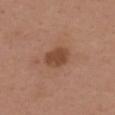Imaged during a routine full-body skin examination; the lesion was not biopsied and no histopathology is available. Imaged with white-light lighting. A male subject aged around 40. Longest diameter approximately 3 mm. A 15 mm close-up tile from a total-body photography series done for melanoma screening. Located on the upper back.